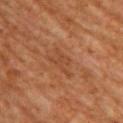Findings:
- biopsy status · catalogued during a skin exam; not biopsied
- patient · female, aged around 65
- site · the back
- acquisition · total-body-photography crop, ~15 mm field of view